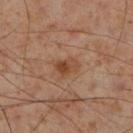Assessment: The lesion was photographed on a routine skin check and not biopsied; there is no pathology result. Context: A 15 mm close-up extracted from a 3D total-body photography capture. A male subject, approximately 55 years of age. Located on the right lower leg.